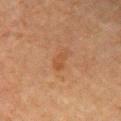notes = catalogued during a skin exam; not biopsied | image = 15 mm crop, total-body photography | diameter = ≈3 mm | patient = male, aged approximately 80 | illumination = cross-polarized | body site = the front of the torso.